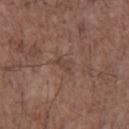| key | value |
|---|---|
| biopsy status | imaged on a skin check; not biopsied |
| anatomic site | the front of the torso |
| patient | male, about 70 years old |
| image | 15 mm crop, total-body photography |